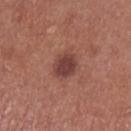notes: catalogued during a skin exam; not biopsied
subject: male, roughly 70 years of age
acquisition: 15 mm crop, total-body photography
body site: the left lower leg
tile lighting: white-light illumination
lesion size: ~3 mm (longest diameter)
automated lesion analysis: a footprint of about 6.5 mm²; an average lesion color of about L≈41 a*≈24 b*≈23 (CIELAB) and about 11 CIELAB-L* units darker than the surrounding skin